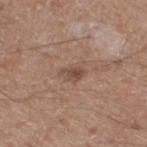Impression: No biopsy was performed on this lesion — it was imaged during a full skin examination and was not determined to be concerning. Context: Captured under white-light illumination. This image is a 15 mm lesion crop taken from a total-body photograph. An algorithmic analysis of the crop reported a symmetry-axis asymmetry near 0.35. And it measured a lesion color around L≈47 a*≈19 b*≈25 in CIELAB and a normalized lesion–skin contrast near 7. And it measured a within-lesion color-variation index near 2/10. The lesion's longest dimension is about 2.5 mm. The patient is a male in their mid-60s. On the right thigh.